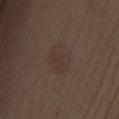Notes:
– notes · no biopsy performed (imaged during a skin exam)
– subject · female, roughly 50 years of age
– acquisition · total-body-photography crop, ~15 mm field of view
– site · the chest
– illumination · white-light illumination
– image-analysis metrics · an area of roughly 10 mm², a shape eccentricity near 0.6, and a symmetry-axis asymmetry near 0.2; about 4 CIELAB-L* units darker than the surrounding skin and a normalized border contrast of about 4
– size · ≈4 mm